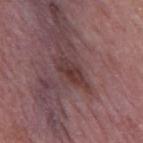notes — no biopsy performed (imaged during a skin exam) | image — 15 mm crop, total-body photography | subject — male, aged 53 to 57 | size — about 3.5 mm | location — the mid back.